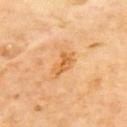| key | value |
|---|---|
| biopsy status | imaged on a skin check; not biopsied |
| illumination | cross-polarized |
| size | ≈3.5 mm |
| acquisition | 15 mm crop, total-body photography |
| anatomic site | the upper back |
| automated metrics | an average lesion color of about L≈66 a*≈25 b*≈48 (CIELAB) and about 9 CIELAB-L* units darker than the surrounding skin; a nevus-likeness score of about 0/100 and a detector confidence of about 100 out of 100 that the crop contains a lesion |